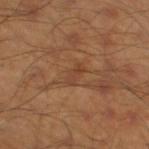Part of a total-body skin-imaging series; this lesion was reviewed on a skin check and was not flagged for biopsy. Cropped from a total-body skin-imaging series; the visible field is about 15 mm. The tile uses cross-polarized illumination. The patient is a male in their mid- to late 40s. From the right thigh. The recorded lesion diameter is about 2.5 mm.